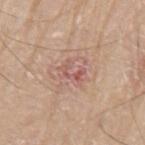Part of a total-body skin-imaging series; this lesion was reviewed on a skin check and was not flagged for biopsy. From the arm. A 15 mm crop from a total-body photograph taken for skin-cancer surveillance. The patient is a male in their 80s. Imaged with white-light lighting.